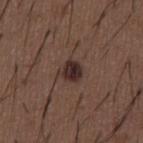Notes:
- workup: total-body-photography surveillance lesion; no biopsy
- automated lesion analysis: an area of roughly 6 mm² and an outline eccentricity of about 0.35 (0 = round, 1 = elongated); a border-irregularity index near 1.5/10, a within-lesion color-variation index near 5.5/10, and radial color variation of about 2
- lighting: white-light illumination
- image source: total-body-photography crop, ~15 mm field of view
- lesion diameter: about 2.5 mm
- patient: male, approximately 50 years of age
- location: the front of the torso